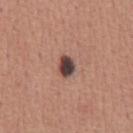Assessment: This lesion was catalogued during total-body skin photography and was not selected for biopsy. Background: A region of skin cropped from a whole-body photographic capture, roughly 15 mm wide. The subject is a male aged 43 to 47. From the chest.